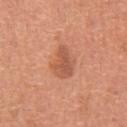{"biopsy_status": "not biopsied; imaged during a skin examination", "patient": {"sex": "male", "age_approx": 65}, "site": "abdomen", "image": {"source": "total-body photography crop", "field_of_view_mm": 15}}